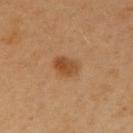Notes:
– follow-up: total-body-photography surveillance lesion; no biopsy
– subject: male, in their 40s
– tile lighting: cross-polarized illumination
– anatomic site: the right upper arm
– size: about 3 mm
– image source: ~15 mm crop, total-body skin-cancer survey